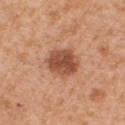Imaged during a routine full-body skin examination; the lesion was not biopsied and no histopathology is available. The recorded lesion diameter is about 4 mm. Cropped from a whole-body photographic skin survey; the tile spans about 15 mm. Imaged with white-light lighting. Located on the chest. A male patient, aged around 55.